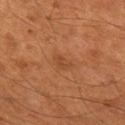Assessment:
Part of a total-body skin-imaging series; this lesion was reviewed on a skin check and was not flagged for biopsy.
Image and clinical context:
A male subject, aged 53 to 57. The total-body-photography lesion software estimated a lesion area of about 3.5 mm² and a shape-asymmetry score of about 0.4 (0 = symmetric). The software also gave a mean CIELAB color near L≈44 a*≈24 b*≈35 and a lesion-to-skin contrast of about 5 (normalized; higher = more distinct). And it measured a border-irregularity index near 3.5/10 and internal color variation of about 2 on a 0–10 scale. A 15 mm crop from a total-body photograph taken for skin-cancer surveillance. The lesion is on the left thigh.